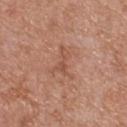This lesion was catalogued during total-body skin photography and was not selected for biopsy. Automated tile analysis of the lesion measured a footprint of about 5 mm², an eccentricity of roughly 0.85, and a symmetry-axis asymmetry near 0.65. It also reported a within-lesion color-variation index near 1.5/10 and a peripheral color-asymmetry measure near 0.5. It also reported an automated nevus-likeness rating near 0 out of 100 and lesion-presence confidence of about 100/100. A female patient, in their mid-70s. A lesion tile, about 15 mm wide, cut from a 3D total-body photograph. About 4 mm across. Imaged with white-light lighting. Located on the upper back.